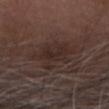Q: What lighting was used for the tile?
A: white-light
Q: What did automated image analysis measure?
A: an area of roughly 15 mm² and an eccentricity of roughly 0.8; a mean CIELAB color near L≈27 a*≈14 b*≈19 and roughly 6 lightness units darker than nearby skin; a nevus-likeness score of about 0/100 and lesion-presence confidence of about 90/100
Q: Patient demographics?
A: male, aged approximately 75
Q: Lesion location?
A: the head or neck
Q: Lesion size?
A: about 6 mm
Q: What is the imaging modality?
A: 15 mm crop, total-body photography